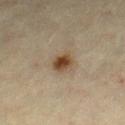Assessment: Captured during whole-body skin photography for melanoma surveillance; the lesion was not biopsied. Clinical summary: About 3.5 mm across. Captured under cross-polarized illumination. A female patient aged approximately 65. This image is a 15 mm lesion crop taken from a total-body photograph. On the right lower leg.